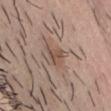biopsy status: catalogued during a skin exam; not biopsied | imaging modality: 15 mm crop, total-body photography | lighting: white-light | site: the head or neck | TBP lesion metrics: a lesion area of about 4.5 mm², a shape eccentricity near 0.55, and a shape-asymmetry score of about 0.5 (0 = symmetric); a nevus-likeness score of about 15/100 | subject: male, approximately 25 years of age | lesion diameter: ≈3 mm.